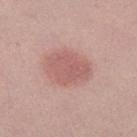patient — female, aged around 20
tile lighting — white-light illumination
location — the right thigh
acquisition — 15 mm crop, total-body photography